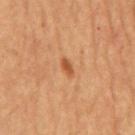Q: Was this lesion biopsied?
A: no biopsy performed (imaged during a skin exam)
Q: What is the anatomic site?
A: the abdomen
Q: What is the imaging modality?
A: ~15 mm tile from a whole-body skin photo
Q: Who is the patient?
A: male, aged 63–67
Q: How was the tile lit?
A: cross-polarized illumination